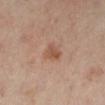No biopsy was performed on this lesion — it was imaged during a full skin examination and was not determined to be concerning. Longest diameter approximately 2.5 mm. Imaged with cross-polarized lighting. A female subject approximately 40 years of age. A 15 mm close-up extracted from a 3D total-body photography capture. On the left leg.